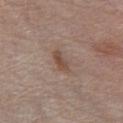follow-up — total-body-photography surveillance lesion; no biopsy
patient — male, in their 80s
diameter — ~3 mm (longest diameter)
anatomic site — the chest
TBP lesion metrics — an eccentricity of roughly 0.9 and a shape-asymmetry score of about 0.3 (0 = symmetric); a lesion color around L≈48 a*≈17 b*≈26 in CIELAB, roughly 9 lightness units darker than nearby skin, and a normalized border contrast of about 7; a border-irregularity index near 3.5/10 and radial color variation of about 0.5; an automated nevus-likeness rating near 20 out of 100 and a lesion-detection confidence of about 100/100
tile lighting — white-light illumination
acquisition — ~15 mm crop, total-body skin-cancer survey